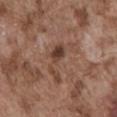Captured during whole-body skin photography for melanoma surveillance; the lesion was not biopsied.
Measured at roughly 6 mm in maximum diameter.
The patient is a male aged approximately 75.
The lesion is on the abdomen.
A lesion tile, about 15 mm wide, cut from a 3D total-body photograph.